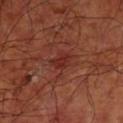Q: Is there a histopathology result?
A: total-body-photography surveillance lesion; no biopsy
Q: How was the tile lit?
A: cross-polarized illumination
Q: What did automated image analysis measure?
A: a lesion area of about 3.5 mm², an eccentricity of roughly 0.7, and two-axis asymmetry of about 0.35; a border-irregularity rating of about 3.5/10, a color-variation rating of about 1/10, and peripheral color asymmetry of about 0.5; an automated nevus-likeness rating near 0 out of 100 and lesion-presence confidence of about 95/100
Q: Lesion location?
A: the left upper arm
Q: What kind of image is this?
A: 15 mm crop, total-body photography
Q: Lesion size?
A: about 2.5 mm
Q: What are the patient's age and sex?
A: male, roughly 65 years of age